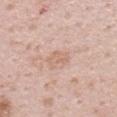This lesion was catalogued during total-body skin photography and was not selected for biopsy. Imaged with white-light lighting. The lesion is located on the upper back. A male subject, in their mid- to late 30s. A roughly 15 mm field-of-view crop from a total-body skin photograph.